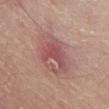Image and clinical context: The recorded lesion diameter is about 3.5 mm. This is a white-light tile. The lesion is located on the abdomen. A male subject aged around 80. Cropped from a whole-body photographic skin survey; the tile spans about 15 mm.